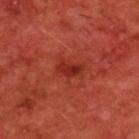No biopsy was performed on this lesion — it was imaged during a full skin examination and was not determined to be concerning. Imaged with cross-polarized lighting. About 3 mm across. A male subject aged 58 to 62. The lesion-visualizer software estimated an average lesion color of about L≈31 a*≈36 b*≈32 (CIELAB), a lesion–skin lightness drop of about 8, and a normalized border contrast of about 7. And it measured a border-irregularity index near 3.5/10 and radial color variation of about 1.5. A 15 mm crop from a total-body photograph taken for skin-cancer surveillance. From the upper back.